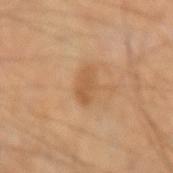A region of skin cropped from a whole-body photographic capture, roughly 15 mm wide. A male subject, aged 58–62. This is a cross-polarized tile. Located on the left forearm. The lesion's longest dimension is about 3.5 mm.